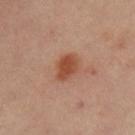<lesion>
  <biopsy_status>not biopsied; imaged during a skin examination</biopsy_status>
  <lesion_size>
    <long_diameter_mm_approx>3.5</long_diameter_mm_approx>
  </lesion_size>
  <image>
    <source>total-body photography crop</source>
    <field_of_view_mm>15</field_of_view_mm>
  </image>
  <automated_metrics>
    <area_mm2_approx>6.0</area_mm2_approx>
    <eccentricity>0.7</eccentricity>
    <shape_asymmetry>0.2</shape_asymmetry>
  </automated_metrics>
  <patient>
    <sex>female</sex>
    <age_approx>40</age_approx>
  </patient>
  <site>leg</site>
</lesion>